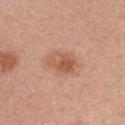biopsy_status: not biopsied; imaged during a skin examination
site: front of the torso
image:
  source: total-body photography crop
  field_of_view_mm: 15
lighting: white-light
lesion_size:
  long_diameter_mm_approx: 4.0
patient:
  sex: female
  age_approx: 30